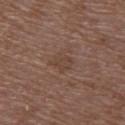Clinical summary: The lesion is located on the upper back. The patient is a female aged around 70. Cropped from a total-body skin-imaging series; the visible field is about 15 mm.